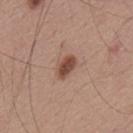Impression:
No biopsy was performed on this lesion — it was imaged during a full skin examination and was not determined to be concerning.
Image and clinical context:
Located on the upper back. A male patient approximately 55 years of age. A 15 mm crop from a total-body photograph taken for skin-cancer surveillance.